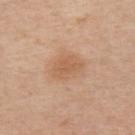| key | value |
|---|---|
| biopsy status | imaged on a skin check; not biopsied |
| TBP lesion metrics | a mean CIELAB color near L≈59 a*≈20 b*≈34; a border-irregularity rating of about 2/10, internal color variation of about 2 on a 0–10 scale, and peripheral color asymmetry of about 0.5; an automated nevus-likeness rating near 5 out of 100 |
| patient | male, roughly 60 years of age |
| imaging modality | total-body-photography crop, ~15 mm field of view |
| body site | the left upper arm |
| diameter | ≈3.5 mm |